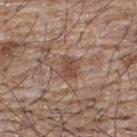notes = imaged on a skin check; not biopsied | patient = male, aged 63–67 | location = the upper back | illumination = white-light | image source = total-body-photography crop, ~15 mm field of view.